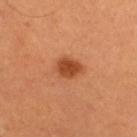  biopsy_status: not biopsied; imaged during a skin examination
  patient:
    sex: male
    age_approx: 50
  site: right thigh
  lighting: cross-polarized
  image:
    source: total-body photography crop
    field_of_view_mm: 15
  lesion_size:
    long_diameter_mm_approx: 3.0